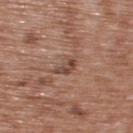Clinical impression:
Recorded during total-body skin imaging; not selected for excision or biopsy.
Clinical summary:
The lesion is on the back. A region of skin cropped from a whole-body photographic capture, roughly 15 mm wide. A male subject aged approximately 50.